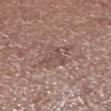{"biopsy_status": "not biopsied; imaged during a skin examination", "patient": {"sex": "male", "age_approx": 75}, "lesion_size": {"long_diameter_mm_approx": 4.5}, "lighting": "white-light", "site": "right lower leg", "image": {"source": "total-body photography crop", "field_of_view_mm": 15}, "automated_metrics": {"cielab_L": 51, "cielab_a": 17, "cielab_b": 22, "vs_skin_darker_L": 6.0, "vs_skin_contrast_norm": 4.5, "border_irregularity_0_10": 3.5, "peripheral_color_asymmetry": 1.5}}